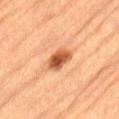- notes — imaged on a skin check; not biopsied
- image-analysis metrics — a footprint of about 6 mm², an outline eccentricity of about 0.6 (0 = round, 1 = elongated), and two-axis asymmetry of about 0.3; border irregularity of about 2.5 on a 0–10 scale and radial color variation of about 1.5
- body site — the lower back
- lighting — cross-polarized illumination
- size — ≈3 mm
- image — total-body-photography crop, ~15 mm field of view
- patient — male, aged 83 or over